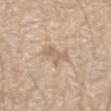Q: Was this lesion biopsied?
A: no biopsy performed (imaged during a skin exam)
Q: Lesion size?
A: about 3.5 mm
Q: What kind of image is this?
A: total-body-photography crop, ~15 mm field of view
Q: Lesion location?
A: the upper back
Q: What are the patient's age and sex?
A: male, in their 80s